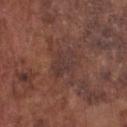Q: Is there a histopathology result?
A: imaged on a skin check; not biopsied
Q: What lighting was used for the tile?
A: white-light
Q: What kind of image is this?
A: 15 mm crop, total-body photography
Q: Who is the patient?
A: male, aged approximately 75
Q: What did automated image analysis measure?
A: a footprint of about 7 mm², a shape eccentricity near 0.75, and a shape-asymmetry score of about 0.25 (0 = symmetric); a mean CIELAB color near L≈35 a*≈19 b*≈20 and a lesion–skin lightness drop of about 5; a within-lesion color-variation index near 2.5/10 and peripheral color asymmetry of about 1; an automated nevus-likeness rating near 0 out of 100 and a detector confidence of about 85 out of 100 that the crop contains a lesion
Q: Lesion location?
A: the chest
Q: How large is the lesion?
A: ≈3.5 mm